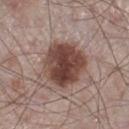Impression: The lesion was photographed on a routine skin check and not biopsied; there is no pathology result. Context: The tile uses white-light illumination. On the leg. A lesion tile, about 15 mm wide, cut from a 3D total-body photograph. Approximately 5.5 mm at its widest. An algorithmic analysis of the crop reported a lesion area of about 22 mm², an outline eccentricity of about 0.3 (0 = round, 1 = elongated), and a shape-asymmetry score of about 0.15 (0 = symmetric). The analysis additionally found a lesion–skin lightness drop of about 15 and a normalized lesion–skin contrast near 11.5. The software also gave a nevus-likeness score of about 85/100 and lesion-presence confidence of about 100/100. The subject is a male approximately 60 years of age.